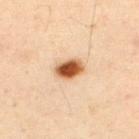Impression: Recorded during total-body skin imaging; not selected for excision or biopsy. Image and clinical context: This is a cross-polarized tile. The lesion's longest dimension is about 3.5 mm. The patient is a male aged approximately 50. On the upper back. A 15 mm crop from a total-body photograph taken for skin-cancer surveillance.